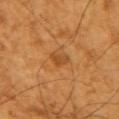The lesion was tiled from a total-body skin photograph and was not biopsied. On the arm. This is a cross-polarized tile. The recorded lesion diameter is about 2.5 mm. A male subject, aged 58–62. A lesion tile, about 15 mm wide, cut from a 3D total-body photograph.